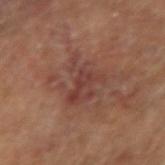Part of a total-body skin-imaging series; this lesion was reviewed on a skin check and was not flagged for biopsy. Measured at roughly 5 mm in maximum diameter. On the right upper arm. Automated image analysis of the tile measured a lesion area of about 11 mm², an eccentricity of roughly 0.6, and a symmetry-axis asymmetry near 0.55. It also reported a lesion-detection confidence of about 95/100. Imaged with cross-polarized lighting. A male subject aged 63–67. A 15 mm close-up tile from a total-body photography series done for melanoma screening.